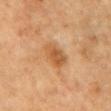<tbp_lesion>
  <biopsy_status>not biopsied; imaged during a skin examination</biopsy_status>
  <lighting>cross-polarized</lighting>
  <site>arm</site>
  <image>
    <source>total-body photography crop</source>
    <field_of_view_mm>15</field_of_view_mm>
  </image>
  <patient>
    <sex>female</sex>
    <age_approx>55</age_approx>
  </patient>
</tbp_lesion>